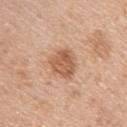workup: no biopsy performed (imaged during a skin exam)
acquisition: ~15 mm crop, total-body skin-cancer survey
patient: male, aged approximately 55
automated metrics: a footprint of about 9.5 mm² and two-axis asymmetry of about 0.25; a peripheral color-asymmetry measure near 1.5
site: the arm
lesion diameter: about 4 mm